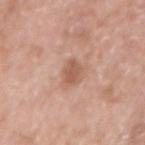A male subject in their 50s. From the right upper arm. A 15 mm crop from a total-body photograph taken for skin-cancer surveillance. This is a white-light tile.